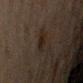Impression: No biopsy was performed on this lesion — it was imaged during a full skin examination and was not determined to be concerning. Image and clinical context: The lesion is located on the arm. A 15 mm crop from a total-body photograph taken for skin-cancer surveillance. Longest diameter approximately 2.5 mm. The tile uses cross-polarized illumination. A male patient aged 58 to 62.